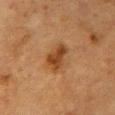Clinical impression:
Recorded during total-body skin imaging; not selected for excision or biopsy.
Background:
A 15 mm close-up tile from a total-body photography series done for melanoma screening. The patient is a female aged around 55. The lesion is located on the chest.